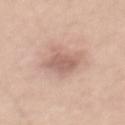Q: Is there a histopathology result?
A: imaged on a skin check; not biopsied
Q: Automated lesion metrics?
A: a mean CIELAB color near L≈62 a*≈19 b*≈25; a nevus-likeness score of about 5/100 and a lesion-detection confidence of about 100/100
Q: Lesion location?
A: the front of the torso
Q: Who is the patient?
A: male, aged 48–52
Q: What is the imaging modality?
A: ~15 mm tile from a whole-body skin photo